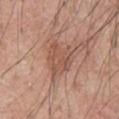* biopsy status · total-body-photography surveillance lesion; no biopsy
* lesion diameter · about 4.5 mm
* location · the chest
* lighting · white-light
* subject · male, approximately 55 years of age
* acquisition · ~15 mm tile from a whole-body skin photo